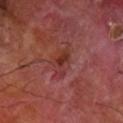The lesion was photographed on a routine skin check and not biopsied; there is no pathology result. Captured under cross-polarized illumination. Automated tile analysis of the lesion measured an average lesion color of about L≈34 a*≈28 b*≈25 (CIELAB), about 7 CIELAB-L* units darker than the surrounding skin, and a lesion-to-skin contrast of about 6.5 (normalized; higher = more distinct). The software also gave border irregularity of about 4.5 on a 0–10 scale and a within-lesion color-variation index near 3/10. A male subject in their 70s. A 15 mm crop from a total-body photograph taken for skin-cancer surveillance. Located on the right forearm. The lesion's longest dimension is about 3.5 mm.